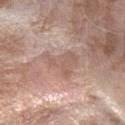image source: 15 mm crop, total-body photography; subject: female, in their mid-70s; illumination: white-light illumination; diameter: about 4.5 mm; location: the right forearm.